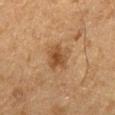This lesion was catalogued during total-body skin photography and was not selected for biopsy.